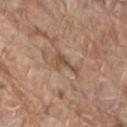Image and clinical context:
The lesion is on the back. A male subject, aged approximately 80. A lesion tile, about 15 mm wide, cut from a 3D total-body photograph.